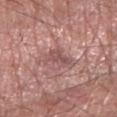Notes:
* biopsy status · imaged on a skin check; not biopsied
* size · about 3 mm
* TBP lesion metrics · a lesion area of about 5.5 mm² and two-axis asymmetry of about 0.4
* anatomic site · the right forearm
* patient · male, aged 58–62
* image source · ~15 mm crop, total-body skin-cancer survey